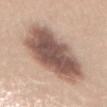Imaged during a routine full-body skin examination; the lesion was not biopsied and no histopathology is available. The subject is a female aged around 45. An algorithmic analysis of the crop reported a lesion area of about 41 mm², a shape eccentricity near 0.85, and a shape-asymmetry score of about 0.15 (0 = symmetric). And it measured a mean CIELAB color near L≈55 a*≈18 b*≈24, a lesion–skin lightness drop of about 17, and a normalized lesion–skin contrast near 11.5. Imaged with white-light lighting. A 15 mm close-up tile from a total-body photography series done for melanoma screening. Approximately 11 mm at its widest. On the mid back.